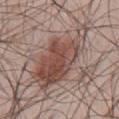No biopsy was performed on this lesion — it was imaged during a full skin examination and was not determined to be concerning. The lesion-visualizer software estimated a mean CIELAB color near L≈48 a*≈19 b*≈23, a lesion–skin lightness drop of about 12, and a normalized lesion–skin contrast near 8.5. And it measured border irregularity of about 4.5 on a 0–10 scale, a color-variation rating of about 6.5/10, and radial color variation of about 2. The software also gave a classifier nevus-likeness of about 95/100 and a lesion-detection confidence of about 100/100. This image is a 15 mm lesion crop taken from a total-body photograph. Imaged with white-light lighting. The lesion is located on the abdomen. A male patient, roughly 50 years of age. The recorded lesion diameter is about 10 mm.